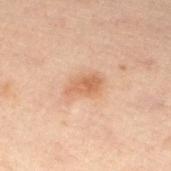Part of a total-body skin-imaging series; this lesion was reviewed on a skin check and was not flagged for biopsy.